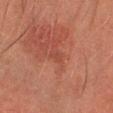acquisition: total-body-photography crop, ~15 mm field of view | TBP lesion metrics: an area of roughly 1.5 mm², a shape eccentricity near 0.75, and a symmetry-axis asymmetry near 0.4; a lesion color around L≈37 a*≈25 b*≈26 in CIELAB, a lesion–skin lightness drop of about 4, and a lesion-to-skin contrast of about 4 (normalized; higher = more distinct); a lesion-detection confidence of about 95/100 | patient: male, aged around 45 | location: the head or neck.